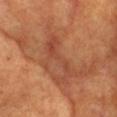patient=female, aged 73 to 77 | image source=~15 mm crop, total-body skin-cancer survey | automated lesion analysis=a border-irregularity index near 9.5/10, a color-variation rating of about 2.5/10, and radial color variation of about 1 | diameter=≈6 mm | body site=the head or neck | lighting=cross-polarized illumination.